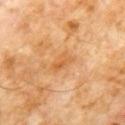Q: Is there a histopathology result?
A: total-body-photography surveillance lesion; no biopsy
Q: Who is the patient?
A: male, approximately 60 years of age
Q: How was this image acquired?
A: ~15 mm crop, total-body skin-cancer survey
Q: How was the tile lit?
A: cross-polarized illumination
Q: Where on the body is the lesion?
A: the chest